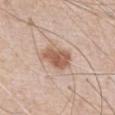follow-up = no biopsy performed (imaged during a skin exam); subject = male, aged 78–82; anatomic site = the abdomen; image source = ~15 mm crop, total-body skin-cancer survey; lighting = white-light.